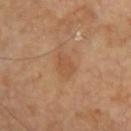biopsy status: catalogued during a skin exam; not biopsied | body site: the right upper arm | patient: male, roughly 60 years of age | image: ~15 mm tile from a whole-body skin photo.